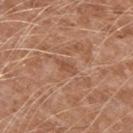Recorded during total-body skin imaging; not selected for excision or biopsy.
Automated image analysis of the tile measured a lesion area of about 3.5 mm², an outline eccentricity of about 0.85 (0 = round, 1 = elongated), and two-axis asymmetry of about 0.35. The analysis additionally found roughly 7 lightness units darker than nearby skin and a normalized lesion–skin contrast near 5. The analysis additionally found internal color variation of about 1.5 on a 0–10 scale.
A male patient in their mid- to late 40s.
Located on the left upper arm.
A region of skin cropped from a whole-body photographic capture, roughly 15 mm wide.
The lesion's longest dimension is about 3 mm.
Captured under white-light illumination.